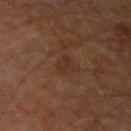biopsy_status: not biopsied; imaged during a skin examination